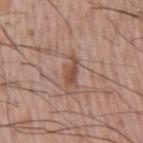The lesion-visualizer software estimated a lesion color around L≈50 a*≈21 b*≈27 in CIELAB. It also reported a detector confidence of about 100 out of 100 that the crop contains a lesion. The lesion is located on the right upper arm. A male subject roughly 55 years of age. Approximately 3.5 mm at its widest. A close-up tile cropped from a whole-body skin photograph, about 15 mm across.